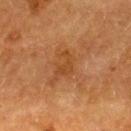<tbp_lesion>
<biopsy_status>not biopsied; imaged during a skin examination</biopsy_status>
<site>back</site>
<patient>
  <sex>female</sex>
  <age_approx>55</age_approx>
</patient>
<automated_metrics>
  <eccentricity>0.8</eccentricity>
  <shape_asymmetry>0.65</shape_asymmetry>
  <border_irregularity_0_10>7.0</border_irregularity_0_10>
  <color_variation_0_10>1.5</color_variation_0_10>
  <peripheral_color_asymmetry>0.5</peripheral_color_asymmetry>
</automated_metrics>
<lesion_size>
  <long_diameter_mm_approx>3.0</long_diameter_mm_approx>
</lesion_size>
<image>
  <source>total-body photography crop</source>
  <field_of_view_mm>15</field_of_view_mm>
</image>
<lighting>cross-polarized</lighting>
</tbp_lesion>